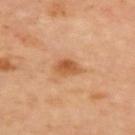The lesion was photographed on a routine skin check and not biopsied; there is no pathology result. On the upper back. This image is a 15 mm lesion crop taken from a total-body photograph. The tile uses cross-polarized illumination.